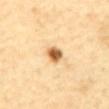workup — no biopsy performed (imaged during a skin exam) | image source — total-body-photography crop, ~15 mm field of view | anatomic site — the front of the torso | size — ~2.5 mm (longest diameter) | subject — male, in their mid- to late 60s | lighting — cross-polarized.